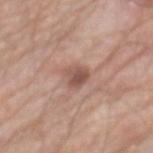workup: catalogued during a skin exam; not biopsied
patient: male, aged approximately 80
lesion diameter: ≈2.5 mm
image source: ~15 mm crop, total-body skin-cancer survey
location: the back
illumination: white-light